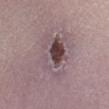Q: Where on the body is the lesion?
A: the left leg
Q: What are the patient's age and sex?
A: female, aged approximately 40
Q: What is the imaging modality?
A: ~15 mm crop, total-body skin-cancer survey